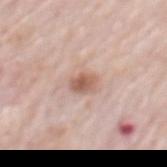<record>
<site>mid back</site>
<patient>
  <sex>male</sex>
  <age_approx>80</age_approx>
</patient>
<image>
  <source>total-body photography crop</source>
  <field_of_view_mm>15</field_of_view_mm>
</image>
</record>